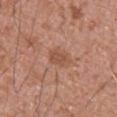The lesion was photographed on a routine skin check and not biopsied; there is no pathology result. A male patient, aged around 75. On the abdomen. The recorded lesion diameter is about 2.5 mm. Cropped from a whole-body photographic skin survey; the tile spans about 15 mm. Imaged with white-light lighting.